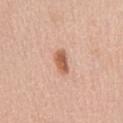Recorded during total-body skin imaging; not selected for excision or biopsy.
Cropped from a total-body skin-imaging series; the visible field is about 15 mm.
The lesion is located on the abdomen.
Longest diameter approximately 3 mm.
This is a white-light tile.
The patient is a male about 60 years old.
The lesion-visualizer software estimated an area of roughly 4.5 mm² and a symmetry-axis asymmetry near 0.2. And it measured border irregularity of about 2 on a 0–10 scale, a color-variation rating of about 2.5/10, and radial color variation of about 1. The software also gave a nevus-likeness score of about 95/100 and a lesion-detection confidence of about 100/100.